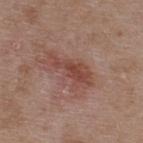notes: catalogued during a skin exam; not biopsied | subject: male, aged 48–52 | acquisition: 15 mm crop, total-body photography | location: the upper back | lesion size: about 6 mm | lighting: white-light.